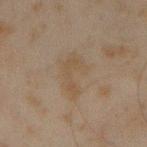No biopsy was performed on this lesion — it was imaged during a full skin examination and was not determined to be concerning. On the right forearm. A 15 mm close-up tile from a total-body photography series done for melanoma screening. The subject is a male approximately 45 years of age. Automated image analysis of the tile measured a lesion area of about 9.5 mm², an eccentricity of roughly 0.85, and two-axis asymmetry of about 0.45. The analysis additionally found a lesion–skin lightness drop of about 4 and a lesion-to-skin contrast of about 5 (normalized; higher = more distinct). It also reported a nevus-likeness score of about 0/100 and a lesion-detection confidence of about 100/100. Captured under cross-polarized illumination.